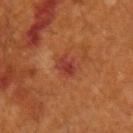Recorded during total-body skin imaging; not selected for excision or biopsy. Approximately 3 mm at its widest. Automated tile analysis of the lesion measured a footprint of about 4.5 mm², an outline eccentricity of about 0.8 (0 = round, 1 = elongated), and two-axis asymmetry of about 0.2. The analysis additionally found an automated nevus-likeness rating near 0 out of 100 and a detector confidence of about 100 out of 100 that the crop contains a lesion. Imaged with cross-polarized lighting. On the right forearm. A region of skin cropped from a whole-body photographic capture, roughly 15 mm wide. The patient is a male aged around 60.